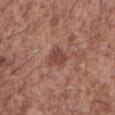  biopsy_status: not biopsied; imaged during a skin examination
  site: abdomen
  image:
    source: total-body photography crop
    field_of_view_mm: 15
  patient:
    sex: male
    age_approx: 45